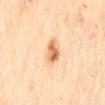| feature | finding |
|---|---|
| biopsy status | catalogued during a skin exam; not biopsied |
| lesion size | about 3.5 mm |
| patient | female, about 60 years old |
| anatomic site | the mid back |
| illumination | cross-polarized |
| image | ~15 mm tile from a whole-body skin photo |